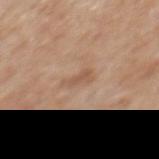Q: Is there a histopathology result?
A: imaged on a skin check; not biopsied
Q: How was this image acquired?
A: ~15 mm crop, total-body skin-cancer survey
Q: What lighting was used for the tile?
A: white-light
Q: What did automated image analysis measure?
A: a lesion color around L≈56 a*≈19 b*≈31 in CIELAB, roughly 7 lightness units darker than nearby skin, and a normalized border contrast of about 5; border irregularity of about 4 on a 0–10 scale, internal color variation of about 1.5 on a 0–10 scale, and a peripheral color-asymmetry measure near 0.5
Q: Who is the patient?
A: male, roughly 60 years of age
Q: Lesion size?
A: about 3 mm
Q: Where on the body is the lesion?
A: the mid back